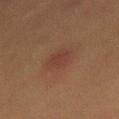The lesion was tiled from a total-body skin photograph and was not biopsied. Captured under cross-polarized illumination. About 3.5 mm across. A female subject, aged around 50. On the mid back. A 15 mm close-up tile from a total-body photography series done for melanoma screening. Automated image analysis of the tile measured an average lesion color of about L≈32 a*≈18 b*≈22 (CIELAB), roughly 5 lightness units darker than nearby skin, and a normalized lesion–skin contrast near 5. The analysis additionally found an automated nevus-likeness rating near 15 out of 100 and a lesion-detection confidence of about 100/100.